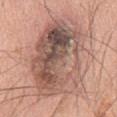The lesion was photographed on a routine skin check and not biopsied; there is no pathology result. A male subject, aged around 60. On the chest. A region of skin cropped from a whole-body photographic capture, roughly 15 mm wide. This is a white-light tile. Measured at roughly 12 mm in maximum diameter. Automated image analysis of the tile measured a classifier nevus-likeness of about 0/100 and a detector confidence of about 100 out of 100 that the crop contains a lesion.